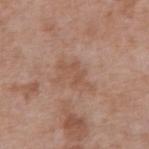Q: Was a biopsy performed?
A: no biopsy performed (imaged during a skin exam)
Q: Illumination type?
A: white-light illumination
Q: Lesion size?
A: about 4.5 mm
Q: What kind of image is this?
A: ~15 mm tile from a whole-body skin photo
Q: What is the anatomic site?
A: the abdomen
Q: What are the patient's age and sex?
A: male, aged around 70